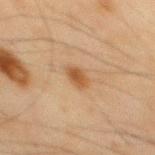| feature | finding |
|---|---|
| biopsy status | total-body-photography surveillance lesion; no biopsy |
| automated metrics | a lesion area of about 4 mm²; an average lesion color of about L≈44 a*≈18 b*≈32 (CIELAB) and about 9 CIELAB-L* units darker than the surrounding skin |
| lesion diameter | ≈2.5 mm |
| anatomic site | the mid back |
| image | ~15 mm crop, total-body skin-cancer survey |
| subject | male, aged 43 to 47 |